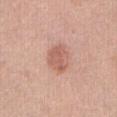{"automated_metrics": {"area_mm2_approx": 8.0, "eccentricity": 0.6, "shape_asymmetry": 0.2, "cielab_L": 60, "cielab_a": 24, "cielab_b": 27, "vs_skin_darker_L": 10.0, "lesion_detection_confidence_0_100": 100}, "lighting": "white-light", "lesion_size": {"long_diameter_mm_approx": 3.5}, "image": {"source": "total-body photography crop", "field_of_view_mm": 15}, "patient": {"sex": "female", "age_approx": 55}, "site": "abdomen"}